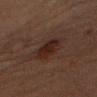Clinical impression: Recorded during total-body skin imaging; not selected for excision or biopsy. Clinical summary: A roughly 15 mm field-of-view crop from a total-body skin photograph. The lesion is located on the right upper arm. A male subject, approximately 60 years of age. The tile uses cross-polarized illumination. The lesion-visualizer software estimated an area of roughly 8.5 mm². The analysis additionally found an average lesion color of about L≈19 a*≈15 b*≈18 (CIELAB), about 6 CIELAB-L* units darker than the surrounding skin, and a lesion-to-skin contrast of about 8.5 (normalized; higher = more distinct). The analysis additionally found a border-irregularity rating of about 2/10, a within-lesion color-variation index near 3/10, and radial color variation of about 1. The analysis additionally found an automated nevus-likeness rating near 70 out of 100 and a lesion-detection confidence of about 100/100. Approximately 4 mm at its widest.